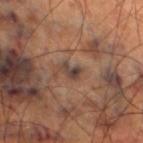biopsy status: catalogued during a skin exam; not biopsied
site: the right thigh
lesion diameter: about 2.5 mm
tile lighting: cross-polarized illumination
patient: male, approximately 70 years of age
imaging modality: ~15 mm tile from a whole-body skin photo
automated metrics: a mean CIELAB color near L≈39 a*≈15 b*≈22 and a lesion–skin lightness drop of about 9; border irregularity of about 3.5 on a 0–10 scale, a color-variation rating of about 2/10, and radial color variation of about 0.5; a detector confidence of about 95 out of 100 that the crop contains a lesion